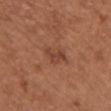The lesion was tiled from a total-body skin photograph and was not biopsied. The tile uses white-light illumination. Automated tile analysis of the lesion measured a footprint of about 5 mm², a shape eccentricity near 0.8, and a shape-asymmetry score of about 0.35 (0 = symmetric). The analysis additionally found radial color variation of about 0.5. From the front of the torso. The subject is a female in their mid-60s. This image is a 15 mm lesion crop taken from a total-body photograph.